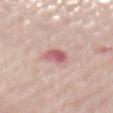Impression: Imaged during a routine full-body skin examination; the lesion was not biopsied and no histopathology is available. Clinical summary: A close-up tile cropped from a whole-body skin photograph, about 15 mm across. Measured at roughly 2.5 mm in maximum diameter. Captured under white-light illumination. The lesion is located on the mid back. A female subject roughly 65 years of age.